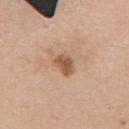Recorded during total-body skin imaging; not selected for excision or biopsy. A female subject in their mid- to late 20s. A lesion tile, about 15 mm wide, cut from a 3D total-body photograph. From the right upper arm.